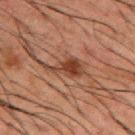Findings:
- biopsy status: no biopsy performed (imaged during a skin exam)